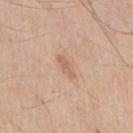notes — catalogued during a skin exam; not biopsied | size — about 3 mm | TBP lesion metrics — an area of roughly 3 mm² and a shape eccentricity near 0.95; a mean CIELAB color near L≈62 a*≈20 b*≈33, about 8 CIELAB-L* units darker than the surrounding skin, and a normalized border contrast of about 6 | acquisition — total-body-photography crop, ~15 mm field of view | illumination — white-light illumination | body site — the chest | subject — male, aged 58 to 62.